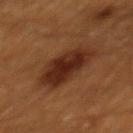Clinical impression:
This lesion was catalogued during total-body skin photography and was not selected for biopsy.
Acquisition and patient details:
From the mid back. Imaged with cross-polarized lighting. Longest diameter approximately 6 mm. A male patient about 60 years old. This image is a 15 mm lesion crop taken from a total-body photograph.